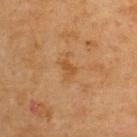follow-up = total-body-photography surveillance lesion; no biopsy | location = the upper back | lighting = cross-polarized illumination | acquisition = 15 mm crop, total-body photography | subject = male, aged 43 to 47.